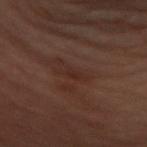| feature | finding |
|---|---|
| notes | imaged on a skin check; not biopsied |
| diameter | ~2.5 mm (longest diameter) |
| image | 15 mm crop, total-body photography |
| automated lesion analysis | a lesion–skin lightness drop of about 4 and a normalized lesion–skin contrast near 5; a within-lesion color-variation index near 1.5/10; a nevus-likeness score of about 0/100 and a detector confidence of about 100 out of 100 that the crop contains a lesion |
| tile lighting | cross-polarized |
| patient | female, aged 58 to 62 |
| anatomic site | the front of the torso |